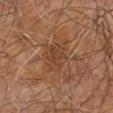| feature | finding |
|---|---|
| biopsy status | total-body-photography surveillance lesion; no biopsy |
| site | the right leg |
| acquisition | ~15 mm crop, total-body skin-cancer survey |
| patient | male, aged 58 to 62 |